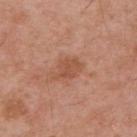Imaged during a routine full-body skin examination; the lesion was not biopsied and no histopathology is available.
This is a white-light tile.
The lesion is on the upper back.
A 15 mm close-up tile from a total-body photography series done for melanoma screening.
The subject is a male approximately 55 years of age.
An algorithmic analysis of the crop reported an outline eccentricity of about 0.7 (0 = round, 1 = elongated) and two-axis asymmetry of about 0.35. It also reported a border-irregularity index near 3.5/10.